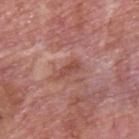<lesion>
<biopsy_status>not biopsied; imaged during a skin examination</biopsy_status>
<lighting>white-light</lighting>
<image>
  <source>total-body photography crop</source>
  <field_of_view_mm>15</field_of_view_mm>
</image>
<automated_metrics>
  <area_mm2_approx>3.5</area_mm2_approx>
  <eccentricity>0.9</eccentricity>
  <shape_asymmetry>0.35</shape_asymmetry>
  <border_irregularity_0_10>4.0</border_irregularity_0_10>
  <color_variation_0_10>1.5</color_variation_0_10>
  <peripheral_color_asymmetry>0.5</peripheral_color_asymmetry>
  <nevus_likeness_0_100>0</nevus_likeness_0_100>
  <lesion_detection_confidence_0_100>85</lesion_detection_confidence_0_100>
</automated_metrics>
<lesion_size>
  <long_diameter_mm_approx>3.0</long_diameter_mm_approx>
</lesion_size>
<patient>
  <sex>male</sex>
  <age_approx>75</age_approx>
</patient>
<site>back</site>
</lesion>